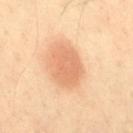This lesion was catalogued during total-body skin photography and was not selected for biopsy. A male patient aged 38 to 42. This is a cross-polarized tile. Located on the right thigh. A roughly 15 mm field-of-view crop from a total-body skin photograph.